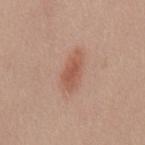The lesion was photographed on a routine skin check and not biopsied; there is no pathology result.
Cropped from a total-body skin-imaging series; the visible field is about 15 mm.
From the mid back.
This is a white-light tile.
Measured at roughly 4.5 mm in maximum diameter.
The patient is a female aged approximately 25.